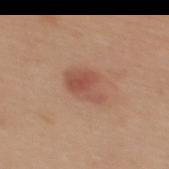– biopsy status — catalogued during a skin exam; not biopsied
– imaging modality — 15 mm crop, total-body photography
– illumination — white-light
– TBP lesion metrics — a lesion area of about 9 mm², an eccentricity of roughly 0.7, and a symmetry-axis asymmetry near 0.25; an average lesion color of about L≈52 a*≈23 b*≈29 (CIELAB), about 9 CIELAB-L* units darker than the surrounding skin, and a normalized border contrast of about 6; a lesion-detection confidence of about 100/100
– site — the upper back
– subject — female, approximately 40 years of age